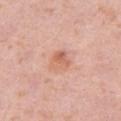Captured during whole-body skin photography for melanoma surveillance; the lesion was not biopsied. A female subject, in their 40s. On the right thigh. A 15 mm close-up tile from a total-body photography series done for melanoma screening.